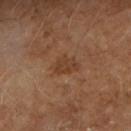Q: Is there a histopathology result?
A: no biopsy performed (imaged during a skin exam)
Q: Where on the body is the lesion?
A: the right forearm
Q: How large is the lesion?
A: ≈3 mm
Q: Illumination type?
A: cross-polarized illumination
Q: What did automated image analysis measure?
A: a lesion area of about 5.5 mm², an outline eccentricity of about 0.7 (0 = round, 1 = elongated), and a symmetry-axis asymmetry near 0.25; a lesion color around L≈38 a*≈19 b*≈30 in CIELAB, about 6 CIELAB-L* units darker than the surrounding skin, and a normalized border contrast of about 6; an automated nevus-likeness rating near 0 out of 100 and lesion-presence confidence of about 100/100
Q: What kind of image is this?
A: 15 mm crop, total-body photography
Q: Patient demographics?
A: male, aged approximately 55